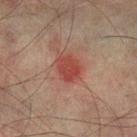Imaged during a routine full-body skin examination; the lesion was not biopsied and no histopathology is available.
This is a cross-polarized tile.
Automated tile analysis of the lesion measured a mean CIELAB color near L≈38 a*≈26 b*≈24, a lesion–skin lightness drop of about 8, and a normalized border contrast of about 7.5. The software also gave a lesion-detection confidence of about 100/100.
About 3.5 mm across.
A lesion tile, about 15 mm wide, cut from a 3D total-body photograph.
A male patient, aged 73–77.
From the left lower leg.